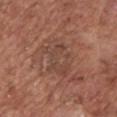follow-up — catalogued during a skin exam; not biopsied
lesion diameter — about 5 mm
patient — male, about 75 years old
imaging modality — 15 mm crop, total-body photography
site — the chest